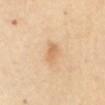<tbp_lesion>
  <image>
    <source>total-body photography crop</source>
    <field_of_view_mm>15</field_of_view_mm>
  </image>
  <patient>
    <sex>female</sex>
    <age_approx>60</age_approx>
  </patient>
  <site>chest</site>
</tbp_lesion>